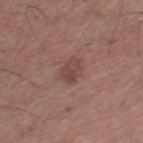Recorded during total-body skin imaging; not selected for excision or biopsy. The lesion is located on the leg. A male patient, roughly 70 years of age. Cropped from a total-body skin-imaging series; the visible field is about 15 mm. Captured under white-light illumination.